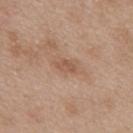Impression: The lesion was tiled from a total-body skin photograph and was not biopsied. Background: A female patient aged 38–42. Measured at roughly 2.5 mm in maximum diameter. A region of skin cropped from a whole-body photographic capture, roughly 15 mm wide. The lesion is on the mid back. The total-body-photography lesion software estimated an area of roughly 3 mm². The software also gave a border-irregularity index near 2.5/10, internal color variation of about 1.5 on a 0–10 scale, and peripheral color asymmetry of about 0.5. And it measured a detector confidence of about 100 out of 100 that the crop contains a lesion. Imaged with white-light lighting.